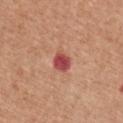{"biopsy_status": "not biopsied; imaged during a skin examination", "site": "chest", "patient": {"sex": "female", "age_approx": 50}, "lesion_size": {"long_diameter_mm_approx": 2.5}, "image": {"source": "total-body photography crop", "field_of_view_mm": 15}}